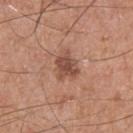<tbp_lesion>
<biopsy_status>not biopsied; imaged during a skin examination</biopsy_status>
<image>
  <source>total-body photography crop</source>
  <field_of_view_mm>15</field_of_view_mm>
</image>
<patient>
  <sex>male</sex>
  <age_approx>55</age_approx>
</patient>
<site>chest</site>
</tbp_lesion>